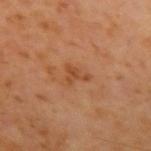<tbp_lesion>
  <biopsy_status>not biopsied; imaged during a skin examination</biopsy_status>
  <patient>
    <sex>male</sex>
    <age_approx>60</age_approx>
  </patient>
  <image>
    <source>total-body photography crop</source>
    <field_of_view_mm>15</field_of_view_mm>
  </image>
  <site>left upper arm</site>
</tbp_lesion>